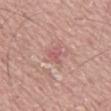follow-up = no biopsy performed (imaged during a skin exam)
imaging modality = total-body-photography crop, ~15 mm field of view
tile lighting = white-light illumination
subject = male, aged approximately 40
image-analysis metrics = a lesion color around L≈57 a*≈26 b*≈22 in CIELAB and a lesion–skin lightness drop of about 7; a classifier nevus-likeness of about 0/100 and lesion-presence confidence of about 85/100
site = the abdomen